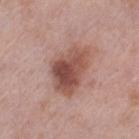Recorded during total-body skin imaging; not selected for excision or biopsy.
A 15 mm close-up tile from a total-body photography series done for melanoma screening.
The patient is a female in their 40s.
Located on the left thigh.